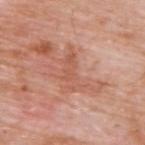Q: What is the imaging modality?
A: ~15 mm tile from a whole-body skin photo
Q: Who is the patient?
A: male, aged around 60
Q: Lesion location?
A: the upper back